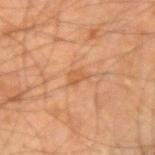Part of a total-body skin-imaging series; this lesion was reviewed on a skin check and was not flagged for biopsy. A male subject aged 63 to 67. The lesion is on the arm. A 15 mm close-up tile from a total-body photography series done for melanoma screening. An algorithmic analysis of the crop reported a lesion area of about 3 mm², an eccentricity of roughly 0.8, and two-axis asymmetry of about 0.35. And it measured a border-irregularity rating of about 3/10, a color-variation rating of about 1/10, and a peripheral color-asymmetry measure near 0.5. The lesion's longest dimension is about 2.5 mm. This is a cross-polarized tile.